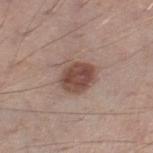{"biopsy_status": "not biopsied; imaged during a skin examination", "site": "right thigh", "patient": {"sex": "male", "age_approx": 55}, "image": {"source": "total-body photography crop", "field_of_view_mm": 15}, "lesion_size": {"long_diameter_mm_approx": 4.0}, "lighting": "white-light", "automated_metrics": {"eccentricity": 0.4, "shape_asymmetry": 0.25, "cielab_L": 48, "cielab_a": 18, "cielab_b": 24, "vs_skin_darker_L": 12.0, "vs_skin_contrast_norm": 9.0, "lesion_detection_confidence_0_100": 100}}